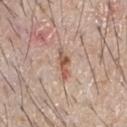Recorded during total-body skin imaging; not selected for excision or biopsy.
The total-body-photography lesion software estimated a lesion color around L≈56 a*≈21 b*≈28 in CIELAB, roughly 11 lightness units darker than nearby skin, and a normalized border contrast of about 8. It also reported a within-lesion color-variation index near 4.5/10 and peripheral color asymmetry of about 1.5. The software also gave a classifier nevus-likeness of about 5/100 and lesion-presence confidence of about 95/100.
A 15 mm close-up tile from a total-body photography series done for melanoma screening.
The lesion is located on the front of the torso.
About 4 mm across.
Captured under white-light illumination.
The patient is a male about 65 years old.